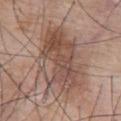Clinical impression: No biopsy was performed on this lesion — it was imaged during a full skin examination and was not determined to be concerning. Acquisition and patient details: The tile uses white-light illumination. A male patient, in their mid-70s. Measured at roughly 8.5 mm in maximum diameter. From the chest. A roughly 15 mm field-of-view crop from a total-body skin photograph. An algorithmic analysis of the crop reported a lesion area of about 30 mm² and two-axis asymmetry of about 0.25. And it measured a lesion–skin lightness drop of about 10 and a normalized lesion–skin contrast near 7. It also reported a classifier nevus-likeness of about 10/100 and lesion-presence confidence of about 100/100.